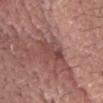The lesion was photographed on a routine skin check and not biopsied; there is no pathology result. Captured under white-light illumination. A male subject, roughly 70 years of age. The total-body-photography lesion software estimated a footprint of about 6.5 mm², an eccentricity of roughly 0.85, and a symmetry-axis asymmetry near 0.25. And it measured lesion-presence confidence of about 65/100. A 15 mm close-up tile from a total-body photography series done for melanoma screening. The lesion is on the head or neck. The recorded lesion diameter is about 4 mm.